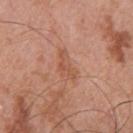Recorded during total-body skin imaging; not selected for excision or biopsy.